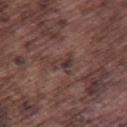The patient is a male aged approximately 75. A 15 mm crop from a total-body photograph taken for skin-cancer surveillance. The tile uses white-light illumination. On the right thigh. The lesion-visualizer software estimated roughly 7 lightness units darker than nearby skin and a normalized border contrast of about 6.5. It also reported an automated nevus-likeness rating near 0 out of 100 and lesion-presence confidence of about 60/100. Measured at roughly 3 mm in maximum diameter.